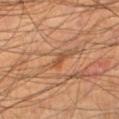No biopsy was performed on this lesion — it was imaged during a full skin examination and was not determined to be concerning. The lesion is located on the right lower leg. A male subject, about 65 years old. A 15 mm crop from a total-body photograph taken for skin-cancer surveillance. Approximately 2 mm at its widest.